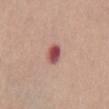Captured during whole-body skin photography for melanoma surveillance; the lesion was not biopsied. From the chest. A female subject, aged around 65. A region of skin cropped from a whole-body photographic capture, roughly 15 mm wide. The tile uses white-light illumination.